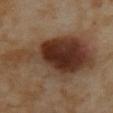| field | value |
|---|---|
| notes | no biopsy performed (imaged during a skin exam) |
| acquisition | total-body-photography crop, ~15 mm field of view |
| body site | the mid back |
| subject | female, in their mid- to late 50s |
| tile lighting | cross-polarized |
| image-analysis metrics | a lesion area of about 46 mm², a shape eccentricity near 0.9, and a symmetry-axis asymmetry near 0.45; a lesion color around L≈36 a*≈18 b*≈27 in CIELAB and roughly 14 lightness units darker than nearby skin; a classifier nevus-likeness of about 100/100 and a detector confidence of about 100 out of 100 that the crop contains a lesion |
| diameter | ≈13 mm |